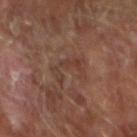workup = imaged on a skin check; not biopsied
imaging modality = ~15 mm crop, total-body skin-cancer survey
diameter = ≈4.5 mm
patient = male, aged around 65
location = the right forearm
tile lighting = cross-polarized illumination
automated lesion analysis = an eccentricity of roughly 0.9; a border-irregularity index near 9.5/10; a nevus-likeness score of about 0/100 and lesion-presence confidence of about 70/100